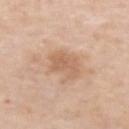This lesion was catalogued during total-body skin photography and was not selected for biopsy.
The patient is a female aged around 55.
Measured at roughly 3.5 mm in maximum diameter.
Automated image analysis of the tile measured a lesion area of about 6 mm² and an outline eccentricity of about 0.7 (0 = round, 1 = elongated). The analysis additionally found a mean CIELAB color near L≈61 a*≈20 b*≈33, roughly 8 lightness units darker than nearby skin, and a normalized lesion–skin contrast near 5.5. The analysis additionally found a border-irregularity index near 4.5/10 and a within-lesion color-variation index near 2/10.
The lesion is on the left forearm.
A 15 mm crop from a total-body photograph taken for skin-cancer surveillance.
Imaged with white-light lighting.